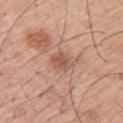Case summary:
- tile lighting · white-light
- body site · the upper back
- acquisition · total-body-photography crop, ~15 mm field of view
- subject · male, aged 53–57